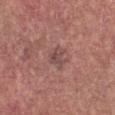Assessment:
This lesion was catalogued during total-body skin photography and was not selected for biopsy.
Acquisition and patient details:
The patient is a female aged approximately 45. The lesion is located on the chest. Cropped from a whole-body photographic skin survey; the tile spans about 15 mm.